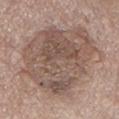Q: Was a biopsy performed?
A: total-body-photography surveillance lesion; no biopsy
Q: Lesion size?
A: about 11 mm
Q: Who is the patient?
A: male, in their 70s
Q: What is the anatomic site?
A: the mid back
Q: Automated lesion metrics?
A: border irregularity of about 3.5 on a 0–10 scale, a within-lesion color-variation index near 6.5/10, and a peripheral color-asymmetry measure near 2.5; a detector confidence of about 100 out of 100 that the crop contains a lesion
Q: Illumination type?
A: white-light illumination
Q: What is the imaging modality?
A: ~15 mm tile from a whole-body skin photo